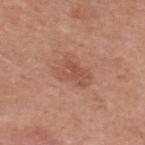workup = catalogued during a skin exam; not biopsied
location = the upper back
lesion diameter = ~4.5 mm (longest diameter)
imaging modality = total-body-photography crop, ~15 mm field of view
patient = male, approximately 50 years of age